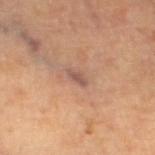Part of a total-body skin-imaging series; this lesion was reviewed on a skin check and was not flagged for biopsy.
About 2.5 mm across.
Located on the left thigh.
Captured under cross-polarized illumination.
A 15 mm close-up extracted from a 3D total-body photography capture.
A male subject aged 58–62.